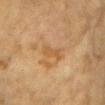This lesion was catalogued during total-body skin photography and was not selected for biopsy.
Longest diameter approximately 3 mm.
The subject is a female aged around 60.
The lesion is located on the chest.
Automated image analysis of the tile measured a lesion area of about 3.5 mm². It also reported a lesion color around L≈52 a*≈18 b*≈38 in CIELAB, about 6 CIELAB-L* units darker than the surrounding skin, and a lesion-to-skin contrast of about 5.5 (normalized; higher = more distinct).
This is a cross-polarized tile.
A 15 mm close-up tile from a total-body photography series done for melanoma screening.